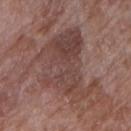Background: A female patient aged approximately 70. An algorithmic analysis of the crop reported a color-variation rating of about 5.5/10 and peripheral color asymmetry of about 2. The analysis additionally found a classifier nevus-likeness of about 0/100 and lesion-presence confidence of about 95/100. A 15 mm crop from a total-body photograph taken for skin-cancer surveillance. The lesion is located on the right upper arm. Imaged with white-light lighting. About 8.5 mm across.